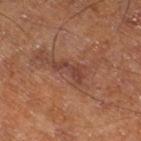workup: catalogued during a skin exam; not biopsied | body site: the leg | subject: male, aged 63–67 | acquisition: 15 mm crop, total-body photography.